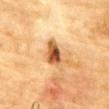automated metrics: a symmetry-axis asymmetry near 0.25; a lesion–skin lightness drop of about 17 and a normalized border contrast of about 11.5; lesion-presence confidence of about 100/100
patient: male, aged around 85 or older
body site: the chest
image source: 15 mm crop, total-body photography
size: ≈3.5 mm